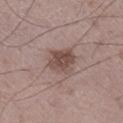The lesion was tiled from a total-body skin photograph and was not biopsied. Approximately 3.5 mm at its widest. This is a white-light tile. On the right lower leg. An algorithmic analysis of the crop reported border irregularity of about 2 on a 0–10 scale and a peripheral color-asymmetry measure near 1.5. The analysis additionally found an automated nevus-likeness rating near 65 out of 100 and lesion-presence confidence of about 100/100. A male subject, about 50 years old. This image is a 15 mm lesion crop taken from a total-body photograph.